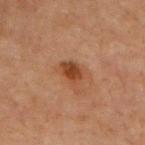Part of a total-body skin-imaging series; this lesion was reviewed on a skin check and was not flagged for biopsy.
Cropped from a total-body skin-imaging series; the visible field is about 15 mm.
A male patient, aged around 70.
Imaged with cross-polarized lighting.
Located on the front of the torso.
The lesion-visualizer software estimated internal color variation of about 2.5 on a 0–10 scale and a peripheral color-asymmetry measure near 1. The software also gave a nevus-likeness score of about 75/100 and a detector confidence of about 100 out of 100 that the crop contains a lesion.
The lesion's longest dimension is about 3 mm.